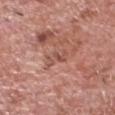notes: no biopsy performed (imaged during a skin exam)
size: ≈2.5 mm
automated metrics: a nevus-likeness score of about 0/100 and lesion-presence confidence of about 90/100
image: ~15 mm tile from a whole-body skin photo
subject: male, aged around 70
body site: the head or neck
illumination: white-light